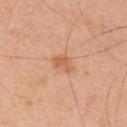Clinical impression: The lesion was tiled from a total-body skin photograph and was not biopsied.